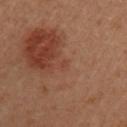<case>
  <biopsy_status>not biopsied; imaged during a skin examination</biopsy_status>
  <image>
    <source>total-body photography crop</source>
    <field_of_view_mm>15</field_of_view_mm>
  </image>
  <site>left upper arm</site>
  <lighting>cross-polarized</lighting>
  <patient>
    <sex>female</sex>
    <age_approx>30</age_approx>
  </patient>
</case>